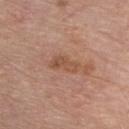workup — total-body-photography surveillance lesion; no biopsy | image source — ~15 mm crop, total-body skin-cancer survey | diameter — ≈4 mm | tile lighting — white-light illumination | location — the chest | patient — male, aged approximately 75.